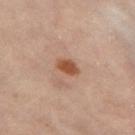Clinical impression:
The lesion was photographed on a routine skin check and not biopsied; there is no pathology result.
Clinical summary:
A lesion tile, about 15 mm wide, cut from a 3D total-body photograph. The lesion is located on the left thigh. Approximately 3 mm at its widest. Captured under cross-polarized illumination. A female patient aged 58–62.